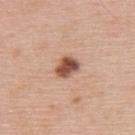Q: Is there a histopathology result?
A: catalogued during a skin exam; not biopsied
Q: How large is the lesion?
A: ≈3 mm
Q: What is the imaging modality?
A: 15 mm crop, total-body photography
Q: What is the anatomic site?
A: the upper back
Q: What lighting was used for the tile?
A: white-light illumination
Q: What are the patient's age and sex?
A: male, in their 60s